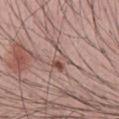notes: no biopsy performed (imaged during a skin exam) | automated lesion analysis: an area of roughly 5 mm², an outline eccentricity of about 0.75 (0 = round, 1 = elongated), and a shape-asymmetry score of about 0.55 (0 = symmetric); a border-irregularity rating of about 6.5/10, a color-variation rating of about 8.5/10, and radial color variation of about 2.5 | illumination: white-light | acquisition: 15 mm crop, total-body photography | patient: male, about 30 years old | anatomic site: the front of the torso | lesion diameter: ~3.5 mm (longest diameter).